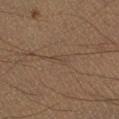biopsy status: imaged on a skin check; not biopsied
diameter: about 2.5 mm
image source: ~15 mm crop, total-body skin-cancer survey
illumination: cross-polarized
anatomic site: the leg
patient: male, aged 38 to 42